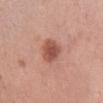Captured during whole-body skin photography for melanoma surveillance; the lesion was not biopsied. From the abdomen. About 3 mm across. The total-body-photography lesion software estimated an eccentricity of roughly 0.55. The analysis additionally found a mean CIELAB color near L≈52 a*≈26 b*≈28 and a normalized lesion–skin contrast near 8.5. It also reported internal color variation of about 3.5 on a 0–10 scale and a peripheral color-asymmetry measure near 1. The subject is a female in their 50s. Cropped from a total-body skin-imaging series; the visible field is about 15 mm.